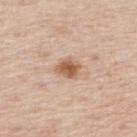Case summary:
- notes: imaged on a skin check; not biopsied
- lighting: white-light
- subject: male, in their mid-70s
- diameter: ~3 mm (longest diameter)
- body site: the upper back
- imaging modality: total-body-photography crop, ~15 mm field of view